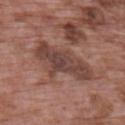A male subject aged 68–72. On the upper back. A 15 mm crop from a total-body photograph taken for skin-cancer surveillance.